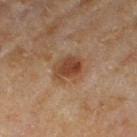Captured during whole-body skin photography for melanoma surveillance; the lesion was not biopsied. A 15 mm close-up tile from a total-body photography series done for melanoma screening. From the right thigh. A female subject, aged 58–62. The tile uses cross-polarized illumination.